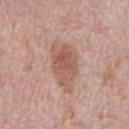Q: Is there a histopathology result?
A: no biopsy performed (imaged during a skin exam)
Q: Where on the body is the lesion?
A: the chest
Q: What is the imaging modality?
A: ~15 mm tile from a whole-body skin photo
Q: Who is the patient?
A: male, aged 68 to 72